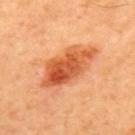Assessment:
This lesion was catalogued during total-body skin photography and was not selected for biopsy.
Image and clinical context:
A close-up tile cropped from a whole-body skin photograph, about 15 mm across. The subject is a male aged 63 to 67. The lesion is located on the back.